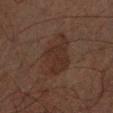From the right forearm. A male patient roughly 50 years of age. The lesion-visualizer software estimated radial color variation of about 1. This image is a 15 mm lesion crop taken from a total-body photograph. The tile uses cross-polarized illumination.